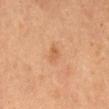The subject is a male about 55 years old. A 15 mm crop from a total-body photograph taken for skin-cancer surveillance. The lesion is located on the mid back.